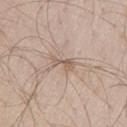Part of a total-body skin-imaging series; this lesion was reviewed on a skin check and was not flagged for biopsy. The lesion-visualizer software estimated a border-irregularity rating of about 4.5/10, internal color variation of about 1 on a 0–10 scale, and peripheral color asymmetry of about 0.5. The subject is a male about 45 years old. Approximately 3 mm at its widest. The lesion is on the leg. A region of skin cropped from a whole-body photographic capture, roughly 15 mm wide. Imaged with white-light lighting.